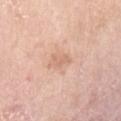Assessment:
Captured during whole-body skin photography for melanoma surveillance; the lesion was not biopsied.
Image and clinical context:
From the left upper arm. Measured at roughly 2.5 mm in maximum diameter. A close-up tile cropped from a whole-body skin photograph, about 15 mm across. A female patient about 70 years old.